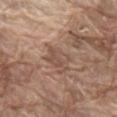No biopsy was performed on this lesion — it was imaged during a full skin examination and was not determined to be concerning.
This image is a 15 mm lesion crop taken from a total-body photograph.
The lesion is on the mid back.
A male patient, aged 78 to 82.
The tile uses white-light illumination.
The recorded lesion diameter is about 3.5 mm.